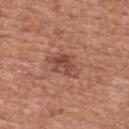{
  "lesion_size": {
    "long_diameter_mm_approx": 4.0
  },
  "patient": {
    "sex": "male",
    "age_approx": 45
  },
  "lighting": "white-light",
  "site": "chest",
  "image": {
    "source": "total-body photography crop",
    "field_of_view_mm": 15
  }
}